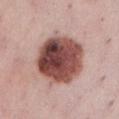workup: imaged on a skin check; not biopsied | anatomic site: the abdomen | lesion size: ≈6.5 mm | patient: female, roughly 25 years of age | acquisition: 15 mm crop, total-body photography.